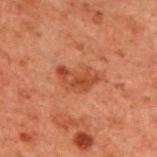Assessment:
No biopsy was performed on this lesion — it was imaged during a full skin examination and was not determined to be concerning.
Background:
The subject is a male aged approximately 60. From the upper back. A close-up tile cropped from a whole-body skin photograph, about 15 mm across.